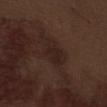biopsy status — no biopsy performed (imaged during a skin exam); site — the abdomen; image — 15 mm crop, total-body photography; patient — male, aged approximately 70.